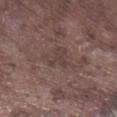No biopsy was performed on this lesion — it was imaged during a full skin examination and was not determined to be concerning.
The recorded lesion diameter is about 3 mm.
A lesion tile, about 15 mm wide, cut from a 3D total-body photograph.
The lesion is on the right lower leg.
A male patient, about 75 years old.
The tile uses white-light illumination.